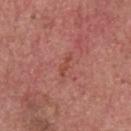Findings:
- follow-up: imaged on a skin check; not biopsied
- lighting: white-light illumination
- size: about 3 mm
- imaging modality: 15 mm crop, total-body photography
- location: the head or neck
- patient: male, about 60 years old